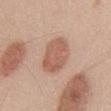The lesion was photographed on a routine skin check and not biopsied; there is no pathology result. About 5.5 mm across. The tile uses white-light illumination. A roughly 15 mm field-of-view crop from a total-body skin photograph. An algorithmic analysis of the crop reported a shape eccentricity near 0.8 and a shape-asymmetry score of about 0.15 (0 = symmetric). The analysis additionally found a mean CIELAB color near L≈59 a*≈22 b*≈29. The patient is a male roughly 50 years of age. The lesion is on the front of the torso.